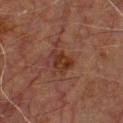Context:
The patient is a male in their 50s. On the chest. The recorded lesion diameter is about 3 mm. The tile uses cross-polarized illumination. Automated tile analysis of the lesion measured a footprint of about 5 mm². The software also gave a classifier nevus-likeness of about 5/100 and a lesion-detection confidence of about 100/100. A 15 mm crop from a total-body photograph taken for skin-cancer surveillance.